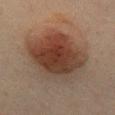| key | value |
|---|---|
| image source | ~15 mm crop, total-body skin-cancer survey |
| patient | female, aged 38 to 42 |
| size | about 9.5 mm |
| lighting | cross-polarized |
| body site | the chest |